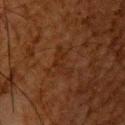The patient is a male approximately 65 years of age. A 15 mm close-up tile from a total-body photography series done for melanoma screening. Imaged with cross-polarized lighting. The lesion is located on the upper back. About 4 mm across.